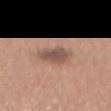Clinical impression:
Imaged during a routine full-body skin examination; the lesion was not biopsied and no histopathology is available.
Clinical summary:
A 15 mm crop from a total-body photograph taken for skin-cancer surveillance. From the abdomen. A female patient aged 38 to 42. Automated tile analysis of the lesion measured a mean CIELAB color near L≈52 a*≈18 b*≈24, a lesion–skin lightness drop of about 11, and a normalized border contrast of about 8.5. The software also gave a color-variation rating of about 4/10 and radial color variation of about 1.5. The software also gave a classifier nevus-likeness of about 55/100 and lesion-presence confidence of about 100/100. About 3.5 mm across. Captured under white-light illumination.